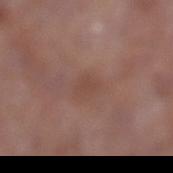notes: no biopsy performed (imaged during a skin exam)
patient: male, aged approximately 55
lesion diameter: ~3 mm (longest diameter)
site: the leg
image: ~15 mm crop, total-body skin-cancer survey
image-analysis metrics: a shape eccentricity near 0.8 and a symmetry-axis asymmetry near 0.35; an average lesion color of about L≈45 a*≈20 b*≈25 (CIELAB) and roughly 6 lightness units darker than nearby skin; internal color variation of about 1 on a 0–10 scale and peripheral color asymmetry of about 0.5